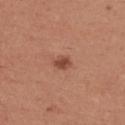Q: Is there a histopathology result?
A: imaged on a skin check; not biopsied
Q: How was this image acquired?
A: ~15 mm crop, total-body skin-cancer survey
Q: What are the patient's age and sex?
A: female, aged 23 to 27
Q: How large is the lesion?
A: about 2.5 mm
Q: What lighting was used for the tile?
A: white-light
Q: What is the anatomic site?
A: the upper back